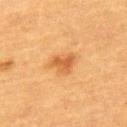notes: catalogued during a skin exam; not biopsied | illumination: cross-polarized | subject: female, aged 53–57 | location: the upper back | imaging modality: ~15 mm tile from a whole-body skin photo | automated metrics: a lesion color around L≈48 a*≈23 b*≈38 in CIELAB and about 9 CIELAB-L* units darker than the surrounding skin.